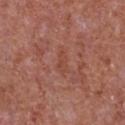Assessment:
Imaged during a routine full-body skin examination; the lesion was not biopsied and no histopathology is available.
Background:
The recorded lesion diameter is about 2.5 mm. A lesion tile, about 15 mm wide, cut from a 3D total-body photograph. Imaged with white-light lighting. On the chest. The lesion-visualizer software estimated a lesion area of about 3 mm², a shape eccentricity near 0.9, and a shape-asymmetry score of about 0.4 (0 = symmetric). And it measured an automated nevus-likeness rating near 0 out of 100 and lesion-presence confidence of about 100/100. The patient is a male about 65 years old.